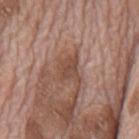Imaged during a routine full-body skin examination; the lesion was not biopsied and no histopathology is available.
The tile uses white-light illumination.
Cropped from a whole-body photographic skin survey; the tile spans about 15 mm.
Located on the back.
Longest diameter approximately 3 mm.
A male subject, aged around 70.